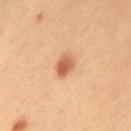No biopsy was performed on this lesion — it was imaged during a full skin examination and was not determined to be concerning. The tile uses cross-polarized illumination. A 15 mm crop from a total-body photograph taken for skin-cancer surveillance. The lesion is located on the left thigh. About 3 mm across. A male subject aged approximately 40.